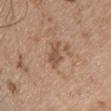Impression:
The lesion was photographed on a routine skin check and not biopsied; there is no pathology result.
Image and clinical context:
A female subject, aged approximately 45. A region of skin cropped from a whole-body photographic capture, roughly 15 mm wide. The tile uses white-light illumination. Located on the upper back. Automated tile analysis of the lesion measured a lesion area of about 4.5 mm², an outline eccentricity of about 0.85 (0 = round, 1 = elongated), and two-axis asymmetry of about 0.35. The software also gave a border-irregularity rating of about 4/10, a within-lesion color-variation index near 1/10, and radial color variation of about 0.5. The lesion's longest dimension is about 3.5 mm.